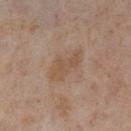No biopsy was performed on this lesion — it was imaged during a full skin examination and was not determined to be concerning.
A 15 mm crop from a total-body photograph taken for skin-cancer surveillance.
Imaged with cross-polarized lighting.
The recorded lesion diameter is about 5 mm.
Located on the right lower leg.
The subject is a female aged 48 to 52.